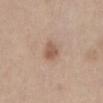Findings:
- workup — imaged on a skin check; not biopsied